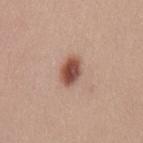This lesion was catalogued during total-body skin photography and was not selected for biopsy. A lesion tile, about 15 mm wide, cut from a 3D total-body photograph. A female subject aged approximately 25. Located on the mid back.